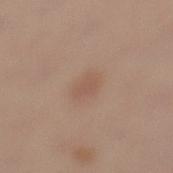The lesion was photographed on a routine skin check and not biopsied; there is no pathology result.
A female patient roughly 40 years of age.
A 15 mm close-up extracted from a 3D total-body photography capture.
Located on the left lower leg.